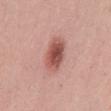follow-up: total-body-photography surveillance lesion; no biopsy | image source: 15 mm crop, total-body photography | automated metrics: a lesion area of about 14 mm², an eccentricity of roughly 0.7, and a symmetry-axis asymmetry near 0.15; a lesion color around L≈56 a*≈24 b*≈25 in CIELAB, about 12 CIELAB-L* units darker than the surrounding skin, and a lesion-to-skin contrast of about 8 (normalized; higher = more distinct); border irregularity of about 1.5 on a 0–10 scale and a within-lesion color-variation index near 6.5/10 | anatomic site: the mid back | size: ≈5 mm | tile lighting: white-light illumination | subject: male, aged 28 to 32.